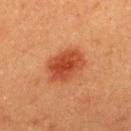  image:
    source: total-body photography crop
    field_of_view_mm: 15
  patient:
    sex: male
    age_approx: 40
  automated_metrics:
    border_irregularity_0_10: 2.0
    color_variation_0_10: 3.5
    peripheral_color_asymmetry: 1.0
  lesion_size:
    long_diameter_mm_approx: 5.0
  site: upper back